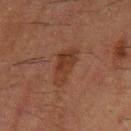The lesion was tiled from a total-body skin photograph and was not biopsied.
The tile uses cross-polarized illumination.
This image is a 15 mm lesion crop taken from a total-body photograph.
The patient is a male aged approximately 50.
The lesion is on the left upper arm.